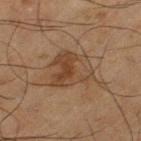Case summary:
- notes · total-body-photography surveillance lesion; no biopsy
- patient · male, aged around 65
- imaging modality · ~15 mm tile from a whole-body skin photo
- anatomic site · the left thigh